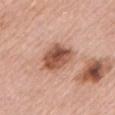Impression: Imaged during a routine full-body skin examination; the lesion was not biopsied and no histopathology is available. Acquisition and patient details: Imaged with white-light lighting. Cropped from a whole-body photographic skin survey; the tile spans about 15 mm. The recorded lesion diameter is about 4.5 mm. The lesion is on the left upper arm. A male patient, roughly 75 years of age.